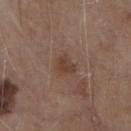Assessment:
Part of a total-body skin-imaging series; this lesion was reviewed on a skin check and was not flagged for biopsy.
Background:
Approximately 2.5 mm at its widest. Cropped from a whole-body photographic skin survey; the tile spans about 15 mm. The total-body-photography lesion software estimated a lesion area of about 4.5 mm², an outline eccentricity of about 0.55 (0 = round, 1 = elongated), and a symmetry-axis asymmetry near 0.3. The software also gave border irregularity of about 3 on a 0–10 scale, internal color variation of about 2.5 on a 0–10 scale, and radial color variation of about 1. Located on the chest. The patient is a male about 80 years old. The tile uses white-light illumination.